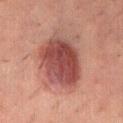Q: Lesion size?
A: ~7 mm (longest diameter)
Q: What lighting was used for the tile?
A: cross-polarized
Q: What is the imaging modality?
A: total-body-photography crop, ~15 mm field of view
Q: Where on the body is the lesion?
A: the abdomen
Q: Automated lesion metrics?
A: an eccentricity of roughly 0.7 and a symmetry-axis asymmetry near 0.1; a mean CIELAB color near L≈42 a*≈24 b*≈22, roughly 13 lightness units darker than nearby skin, and a normalized lesion–skin contrast near 10; a border-irregularity rating of about 1.5/10, internal color variation of about 5.5 on a 0–10 scale, and peripheral color asymmetry of about 1.5; a classifier nevus-likeness of about 85/100 and a lesion-detection confidence of about 100/100
Q: Patient demographics?
A: female, aged 58–62